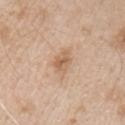Q: Was this lesion biopsied?
A: no biopsy performed (imaged during a skin exam)
Q: How was this image acquired?
A: total-body-photography crop, ~15 mm field of view
Q: Who is the patient?
A: male, about 65 years old
Q: Lesion location?
A: the left upper arm